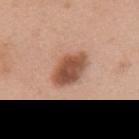A close-up tile cropped from a whole-body skin photograph, about 15 mm across.
From the mid back.
An algorithmic analysis of the crop reported a shape eccentricity near 0.8 and a shape-asymmetry score of about 0.15 (0 = symmetric). And it measured border irregularity of about 1.5 on a 0–10 scale, a color-variation rating of about 4.5/10, and peripheral color asymmetry of about 1.5. The analysis additionally found an automated nevus-likeness rating near 85 out of 100.
A female patient, roughly 50 years of age.